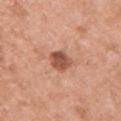This lesion was catalogued during total-body skin photography and was not selected for biopsy. A region of skin cropped from a whole-body photographic capture, roughly 15 mm wide. A female patient, roughly 50 years of age. Imaged with white-light lighting. The lesion's longest dimension is about 3 mm. On the left upper arm.